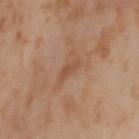patient: female, aged 53 to 57; anatomic site: the left thigh; imaging modality: ~15 mm tile from a whole-body skin photo; lesion size: ≈3 mm; lighting: cross-polarized illumination.